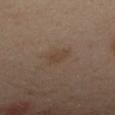Assessment: Imaged during a routine full-body skin examination; the lesion was not biopsied and no histopathology is available. Acquisition and patient details: The lesion is on the left arm. Captured under cross-polarized illumination. Longest diameter approximately 3 mm. The subject is a female aged 38 to 42. A 15 mm close-up tile from a total-body photography series done for melanoma screening.